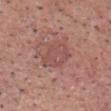Context:
The recorded lesion diameter is about 4 mm. Imaged with white-light lighting. The lesion-visualizer software estimated an area of roughly 9.5 mm², a shape eccentricity near 0.6, and a symmetry-axis asymmetry near 0.15. The software also gave radial color variation of about 1. It also reported an automated nevus-likeness rating near 0 out of 100 and lesion-presence confidence of about 100/100. A male patient, aged 58–62. A 15 mm close-up extracted from a 3D total-body photography capture. The lesion is located on the head or neck.